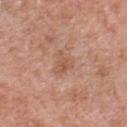{
  "biopsy_status": "not biopsied; imaged during a skin examination",
  "site": "chest",
  "lesion_size": {
    "long_diameter_mm_approx": 3.0
  },
  "image": {
    "source": "total-body photography crop",
    "field_of_view_mm": 15
  },
  "lighting": "white-light",
  "automated_metrics": {
    "area_mm2_approx": 5.0,
    "eccentricity": 0.65,
    "shape_asymmetry": 0.45,
    "cielab_L": 55,
    "cielab_a": 22,
    "cielab_b": 30,
    "vs_skin_contrast_norm": 5.5
  },
  "patient": {
    "sex": "male",
    "age_approx": 70
  }
}